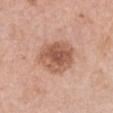Imaged during a routine full-body skin examination; the lesion was not biopsied and no histopathology is available. A female subject, aged 58 to 62. A 15 mm close-up tile from a total-body photography series done for melanoma screening. The lesion is on the chest. The recorded lesion diameter is about 5.5 mm. This is a white-light tile.